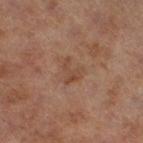biopsy status: imaged on a skin check; not biopsied
location: the right thigh
automated metrics: a footprint of about 4.5 mm², a shape eccentricity near 0.65, and a shape-asymmetry score of about 0.55 (0 = symmetric); a mean CIELAB color near L≈39 a*≈17 b*≈26 and a normalized border contrast of about 5.5; a border-irregularity rating of about 6/10, a color-variation rating of about 1.5/10, and radial color variation of about 0.5; an automated nevus-likeness rating near 0 out of 100 and a lesion-detection confidence of about 100/100
image: ~15 mm crop, total-body skin-cancer survey
tile lighting: cross-polarized
lesion size: ~3 mm (longest diameter)
subject: female, aged approximately 60